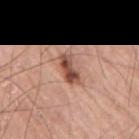Q: Is there a histopathology result?
A: no biopsy performed (imaged during a skin exam)
Q: What are the patient's age and sex?
A: male, aged 73–77
Q: What did automated image analysis measure?
A: a lesion color around L≈50 a*≈23 b*≈28 in CIELAB, a lesion–skin lightness drop of about 15, and a normalized border contrast of about 10
Q: What kind of image is this?
A: 15 mm crop, total-body photography
Q: What is the anatomic site?
A: the right upper arm